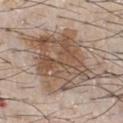This lesion was catalogued during total-body skin photography and was not selected for biopsy. A male subject about 65 years old. The lesion is on the chest. The total-body-photography lesion software estimated a lesion area of about 47 mm², an eccentricity of roughly 0.65, and a shape-asymmetry score of about 0.25 (0 = symmetric). The software also gave an average lesion color of about L≈53 a*≈15 b*≈27 (CIELAB), a lesion–skin lightness drop of about 12, and a normalized lesion–skin contrast near 9. It also reported a border-irregularity rating of about 4.5/10, a within-lesion color-variation index near 7/10, and a peripheral color-asymmetry measure near 2.5. Captured under white-light illumination. A lesion tile, about 15 mm wide, cut from a 3D total-body photograph. The recorded lesion diameter is about 11 mm.